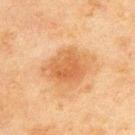biopsy_status: not biopsied; imaged during a skin examination
site: upper back
lesion_size:
  long_diameter_mm_approx: 5.5
automated_metrics:
  area_mm2_approx: 16.0
  shape_asymmetry: 0.2
  nevus_likeness_0_100: 55
  lesion_detection_confidence_0_100: 100
image:
  source: total-body photography crop
  field_of_view_mm: 15
lighting: cross-polarized
patient:
  sex: male
  age_approx: 45